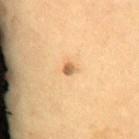Captured under cross-polarized illumination. The lesion is located on the front of the torso. A female subject roughly 65 years of age. A region of skin cropped from a whole-body photographic capture, roughly 15 mm wide. The lesion-visualizer software estimated a lesion color around L≈63 a*≈19 b*≈41 in CIELAB, a lesion–skin lightness drop of about 13, and a normalized lesion–skin contrast near 8. The software also gave a lesion-detection confidence of about 100/100. Approximately 1.5 mm at its widest.